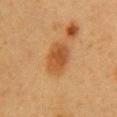No biopsy was performed on this lesion — it was imaged during a full skin examination and was not determined to be concerning. The subject is a female roughly 40 years of age. A close-up tile cropped from a whole-body skin photograph, about 15 mm across. Located on the front of the torso.